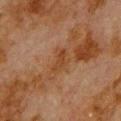Case summary:
- follow-up: catalogued during a skin exam; not biopsied
- body site: the upper back
- patient: male, about 80 years old
- illumination: cross-polarized
- image: 15 mm crop, total-body photography